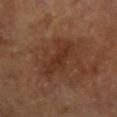This lesion was catalogued during total-body skin photography and was not selected for biopsy.
A female subject in their 80s.
The lesion is located on the right forearm.
The lesion's longest dimension is about 5.5 mm.
A 15 mm crop from a total-body photograph taken for skin-cancer surveillance.